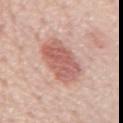Clinical impression: The lesion was photographed on a routine skin check and not biopsied; there is no pathology result. Clinical summary: A male subject about 70 years old. The tile uses white-light illumination. This image is a 15 mm lesion crop taken from a total-body photograph. The lesion-visualizer software estimated a shape eccentricity near 0.85 and a symmetry-axis asymmetry near 0.2. The analysis additionally found a lesion–skin lightness drop of about 12 and a normalized lesion–skin contrast near 7.5. It also reported border irregularity of about 2 on a 0–10 scale and a color-variation rating of about 4/10. It also reported a nevus-likeness score of about 95/100 and lesion-presence confidence of about 100/100. The lesion is located on the chest.